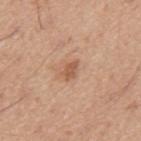Acquisition and patient details: Located on the mid back. Cropped from a total-body skin-imaging series; the visible field is about 15 mm. A male patient, approximately 45 years of age. The lesion's longest dimension is about 2.5 mm. The tile uses white-light illumination.